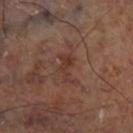Case summary:
- follow-up — total-body-photography surveillance lesion; no biopsy
- automated lesion analysis — a classifier nevus-likeness of about 0/100 and a lesion-detection confidence of about 100/100
- imaging modality — ~15 mm tile from a whole-body skin photo
- tile lighting — cross-polarized illumination
- site — the leg